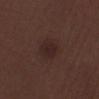Impression:
Imaged during a routine full-body skin examination; the lesion was not biopsied and no histopathology is available.
Clinical summary:
A male subject roughly 70 years of age. The lesion is on the right lower leg. Cropped from a total-body skin-imaging series; the visible field is about 15 mm. Approximately 3 mm at its widest.